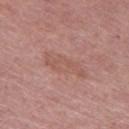No biopsy was performed on this lesion — it was imaged during a full skin examination and was not determined to be concerning. Measured at roughly 5.5 mm in maximum diameter. From the left thigh. A female patient, in their mid- to late 60s. Imaged with white-light lighting. The lesion-visualizer software estimated an average lesion color of about L≈55 a*≈22 b*≈26 (CIELAB), a lesion–skin lightness drop of about 6, and a normalized lesion–skin contrast near 4.5. A roughly 15 mm field-of-view crop from a total-body skin photograph.